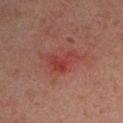  biopsy_status: not biopsied; imaged during a skin examination
  lighting: cross-polarized
  patient:
    sex: male
    age_approx: 30
  image:
    source: total-body photography crop
    field_of_view_mm: 15
  lesion_size:
    long_diameter_mm_approx: 4.0
  automated_metrics:
    area_mm2_approx: 5.5
    eccentricity: 0.8
    shape_asymmetry: 0.6
  site: left upper arm